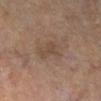The lesion was tiled from a total-body skin photograph and was not biopsied.
The subject is a male in their mid- to late 60s.
On the right lower leg.
The total-body-photography lesion software estimated an automated nevus-likeness rating near 0 out of 100.
Cropped from a total-body skin-imaging series; the visible field is about 15 mm.
About 3 mm across.
The tile uses cross-polarized illumination.